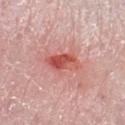Q: Was a biopsy performed?
A: no biopsy performed (imaged during a skin exam)
Q: What is the anatomic site?
A: the right lower leg
Q: What are the patient's age and sex?
A: female, roughly 60 years of age
Q: What kind of image is this?
A: total-body-photography crop, ~15 mm field of view